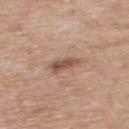Imaged during a routine full-body skin examination; the lesion was not biopsied and no histopathology is available.
Approximately 3.5 mm at its widest.
The lesion is on the back.
A male subject, aged 48 to 52.
A 15 mm close-up tile from a total-body photography series done for melanoma screening.
Captured under white-light illumination.